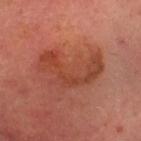Q: Was a biopsy performed?
A: no biopsy performed (imaged during a skin exam)
Q: What is the imaging modality?
A: ~15 mm tile from a whole-body skin photo
Q: What lighting was used for the tile?
A: cross-polarized illumination
Q: What are the patient's age and sex?
A: female, roughly 40 years of age
Q: Lesion size?
A: ~7 mm (longest diameter)
Q: What is the anatomic site?
A: the chest